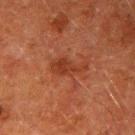Captured during whole-body skin photography for melanoma surveillance; the lesion was not biopsied. Located on the right upper arm. This is a cross-polarized tile. The patient is a male in their 60s. Approximately 4.5 mm at its widest. A lesion tile, about 15 mm wide, cut from a 3D total-body photograph.